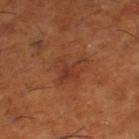Findings:
* follow-up: imaged on a skin check; not biopsied
* subject: male, aged 63–67
* acquisition: ~15 mm tile from a whole-body skin photo
* image-analysis metrics: a classifier nevus-likeness of about 0/100 and a detector confidence of about 100 out of 100 that the crop contains a lesion
* lesion size: ~3.5 mm (longest diameter)
* illumination: cross-polarized illumination
* body site: the right lower leg